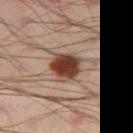notes: catalogued during a skin exam; not biopsied | anatomic site: the right thigh | acquisition: total-body-photography crop, ~15 mm field of view | lesion diameter: about 4 mm | patient: male, aged around 40 | lighting: cross-polarized.